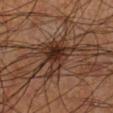Clinical impression:
No biopsy was performed on this lesion — it was imaged during a full skin examination and was not determined to be concerning.
Acquisition and patient details:
The subject is a male aged approximately 60. A 15 mm close-up extracted from a 3D total-body photography capture. From the right lower leg.